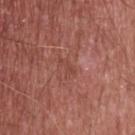{"biopsy_status": "not biopsied; imaged during a skin examination", "lighting": "white-light", "site": "upper back", "lesion_size": {"long_diameter_mm_approx": 2.5}, "patient": {"sex": "male", "age_approx": 55}, "image": {"source": "total-body photography crop", "field_of_view_mm": 15}}